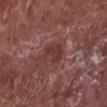This lesion was catalogued during total-body skin photography and was not selected for biopsy.